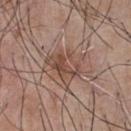No biopsy was performed on this lesion — it was imaged during a full skin examination and was not determined to be concerning.
Located on the chest.
An algorithmic analysis of the crop reported a mean CIELAB color near L≈48 a*≈18 b*≈26, roughly 9 lightness units darker than nearby skin, and a normalized lesion–skin contrast near 6.5. The analysis additionally found an automated nevus-likeness rating near 5 out of 100 and lesion-presence confidence of about 100/100.
Captured under white-light illumination.
A close-up tile cropped from a whole-body skin photograph, about 15 mm across.
A male subject, roughly 55 years of age.
About 4 mm across.